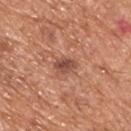biopsy_status: not biopsied; imaged during a skin examination
patient:
  sex: male
  age_approx: 65
lesion_size:
  long_diameter_mm_approx: 2.5
site: upper back
image:
  source: total-body photography crop
  field_of_view_mm: 15
lighting: white-light